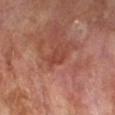{"biopsy_status": "not biopsied; imaged during a skin examination", "lighting": "cross-polarized", "lesion_size": {"long_diameter_mm_approx": 2.5}, "image": {"source": "total-body photography crop", "field_of_view_mm": 15}, "site": "right forearm", "patient": {"sex": "male", "age_approx": 70}}